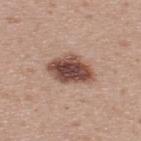Recorded during total-body skin imaging; not selected for excision or biopsy. Measured at roughly 5 mm in maximum diameter. This is a white-light tile. From the upper back. A male patient approximately 35 years of age. A lesion tile, about 15 mm wide, cut from a 3D total-body photograph.